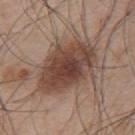Case summary:
• subject: male, in their mid- to late 50s
• image: ~15 mm tile from a whole-body skin photo
• lighting: white-light illumination
• size: ~8 mm (longest diameter)
• automated metrics: a footprint of about 29 mm² and a symmetry-axis asymmetry near 0.15; border irregularity of about 2.5 on a 0–10 scale, a color-variation rating of about 5.5/10, and a peripheral color-asymmetry measure near 1.5; a classifier nevus-likeness of about 70/100
• body site: the upper back